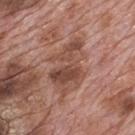<tbp_lesion>
  <biopsy_status>not biopsied; imaged during a skin examination</biopsy_status>
  <automated_metrics>
    <cielab_L>47</cielab_L>
    <cielab_a>22</cielab_a>
    <cielab_b>27</cielab_b>
    <vs_skin_darker_L>10.0</vs_skin_darker_L>
    <vs_skin_contrast_norm>7.5</vs_skin_contrast_norm>
    <nevus_likeness_0_100>0</nevus_likeness_0_100>
  </automated_metrics>
  <image>
    <source>total-body photography crop</source>
    <field_of_view_mm>15</field_of_view_mm>
  </image>
  <lesion_size>
    <long_diameter_mm_approx>6.5</long_diameter_mm_approx>
  </lesion_size>
  <patient>
    <sex>male</sex>
    <age_approx>70</age_approx>
  </patient>
  <site>mid back</site>
</tbp_lesion>